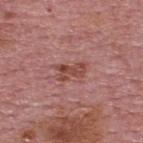{"biopsy_status": "not biopsied; imaged during a skin examination", "site": "upper back", "patient": {"sex": "male", "age_approx": 75}, "automated_metrics": {"border_irregularity_0_10": 4.5, "color_variation_0_10": 1.5, "peripheral_color_asymmetry": 0.5, "nevus_likeness_0_100": 0}, "image": {"source": "total-body photography crop", "field_of_view_mm": 15}, "lesion_size": {"long_diameter_mm_approx": 3.0}, "lighting": "white-light"}